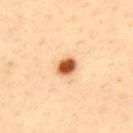On the upper back.
A close-up tile cropped from a whole-body skin photograph, about 15 mm across.
A male patient, aged 38 to 42.
The recorded lesion diameter is about 2.5 mm.